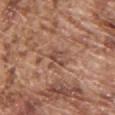{
  "biopsy_status": "not biopsied; imaged during a skin examination",
  "site": "chest",
  "lesion_size": {
    "long_diameter_mm_approx": 2.5
  },
  "patient": {
    "sex": "male",
    "age_approx": 75
  },
  "lighting": "white-light",
  "image": {
    "source": "total-body photography crop",
    "field_of_view_mm": 15
  }
}